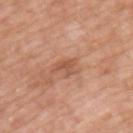Assessment:
Captured during whole-body skin photography for melanoma surveillance; the lesion was not biopsied.
Context:
Longest diameter approximately 2.5 mm. A male subject, roughly 50 years of age. A region of skin cropped from a whole-body photographic capture, roughly 15 mm wide. Located on the upper back.